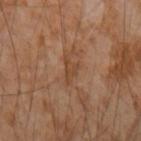Q: What is the anatomic site?
A: the right forearm
Q: Who is the patient?
A: male, aged around 55
Q: What is the imaging modality?
A: total-body-photography crop, ~15 mm field of view
Q: How was the tile lit?
A: cross-polarized illumination
Q: What is the lesion's diameter?
A: ~3 mm (longest diameter)